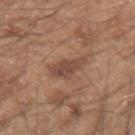The lesion was photographed on a routine skin check and not biopsied; there is no pathology result. A male patient, approximately 50 years of age. Measured at roughly 4 mm in maximum diameter. A close-up tile cropped from a whole-body skin photograph, about 15 mm across. Located on the left forearm.